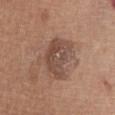Captured during whole-body skin photography for melanoma surveillance; the lesion was not biopsied.
Approximately 5.5 mm at its widest.
A female patient in their mid-50s.
Located on the chest.
Automated image analysis of the tile measured an eccentricity of roughly 0.65 and a symmetry-axis asymmetry near 0.3.
This is a white-light tile.
A close-up tile cropped from a whole-body skin photograph, about 15 mm across.